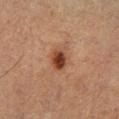<tbp_lesion>
<biopsy_status>not biopsied; imaged during a skin examination</biopsy_status>
<automated_metrics>
  <area_mm2_approx>5.5</area_mm2_approx>
  <eccentricity>0.45</eccentricity>
  <shape_asymmetry>0.15</shape_asymmetry>
  <cielab_L>35</cielab_L>
  <cielab_a>21</cielab_a>
  <cielab_b>27</cielab_b>
  <vs_skin_darker_L>12.0</vs_skin_darker_L>
  <border_irregularity_0_10>1.0</border_irregularity_0_10>
  <color_variation_0_10>4.5</color_variation_0_10>
  <peripheral_color_asymmetry>1.5</peripheral_color_asymmetry>
  <nevus_likeness_0_100>95</nevus_likeness_0_100>
  <lesion_detection_confidence_0_100>100</lesion_detection_confidence_0_100>
</automated_metrics>
<image>
  <source>total-body photography crop</source>
  <field_of_view_mm>15</field_of_view_mm>
</image>
<site>left lower leg</site>
<patient>
  <sex>female</sex>
  <age_approx>40</age_approx>
</patient>
<lesion_size>
  <long_diameter_mm_approx>2.5</long_diameter_mm_approx>
</lesion_size>
</tbp_lesion>